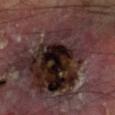The lesion was photographed on a routine skin check and not biopsied; there is no pathology result.
A male subject, aged 68–72.
Automated image analysis of the tile measured a lesion area of about 75 mm², a shape eccentricity near 0.8, and two-axis asymmetry of about 0.4. The analysis additionally found a mean CIELAB color near L≈24 a*≈15 b*≈14, about 12 CIELAB-L* units darker than the surrounding skin, and a normalized lesion–skin contrast near 13. The analysis additionally found internal color variation of about 10 on a 0–10 scale and peripheral color asymmetry of about 4.5. The analysis additionally found a nevus-likeness score of about 0/100 and lesion-presence confidence of about 80/100.
A region of skin cropped from a whole-body photographic capture, roughly 15 mm wide.
Located on the leg.
Approximately 14.5 mm at its widest.